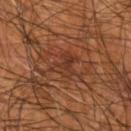The lesion was photographed on a routine skin check and not biopsied; there is no pathology result. Captured under cross-polarized illumination. A male patient, about 55 years old. The lesion's longest dimension is about 3.5 mm. Automated tile analysis of the lesion measured a mean CIELAB color near L≈32 a*≈22 b*≈28, about 5 CIELAB-L* units darker than the surrounding skin, and a lesion-to-skin contrast of about 5.5 (normalized; higher = more distinct). The analysis additionally found border irregularity of about 5 on a 0–10 scale, a color-variation rating of about 3.5/10, and radial color variation of about 1. The analysis additionally found a nevus-likeness score of about 0/100. This image is a 15 mm lesion crop taken from a total-body photograph. The lesion is located on the right forearm.